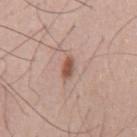{"biopsy_status": "not biopsied; imaged during a skin examination", "lighting": "white-light", "lesion_size": {"long_diameter_mm_approx": 3.0}, "image": {"source": "total-body photography crop", "field_of_view_mm": 15}, "site": "mid back", "patient": {"sex": "male", "age_approx": 50}}